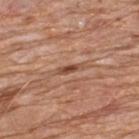<tbp_lesion>
<biopsy_status>not biopsied; imaged during a skin examination</biopsy_status>
<patient>
  <sex>male</sex>
  <age_approx>80</age_approx>
</patient>
<image>
  <source>total-body photography crop</source>
  <field_of_view_mm>15</field_of_view_mm>
</image>
<site>back</site>
<lighting>white-light</lighting>
<automated_metrics>
  <border_irregularity_0_10>3.0</border_irregularity_0_10>
  <color_variation_0_10>0.0</color_variation_0_10>
</automated_metrics>
</tbp_lesion>